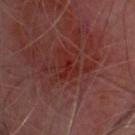The lesion was photographed on a routine skin check and not biopsied; there is no pathology result. A male subject about 60 years old. A lesion tile, about 15 mm wide, cut from a 3D total-body photograph. The lesion is located on the head or neck.